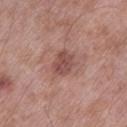Case summary:
* workup · imaged on a skin check; not biopsied
* patient · male, aged 53–57
* lesion size · about 3 mm
* imaging modality · ~15 mm crop, total-body skin-cancer survey
* lighting · white-light
* anatomic site · the left lower leg